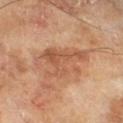workup=catalogued during a skin exam; not biopsied | subject=male, approximately 65 years of age | body site=the right lower leg | image=~15 mm crop, total-body skin-cancer survey.